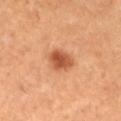| key | value |
|---|---|
| image source | ~15 mm tile from a whole-body skin photo |
| patient | female, in their 30s |
| diameter | ~3.5 mm (longest diameter) |
| anatomic site | the left lower leg |
| tile lighting | cross-polarized |